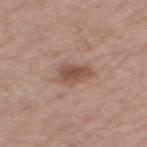notes: total-body-photography surveillance lesion; no biopsy | anatomic site: the left thigh | lighting: white-light illumination | image source: total-body-photography crop, ~15 mm field of view | patient: female, in their mid-60s | automated lesion analysis: an eccentricity of roughly 0.75 and two-axis asymmetry of about 0.25; a lesion color around L≈51 a*≈20 b*≈26 in CIELAB, a lesion–skin lightness drop of about 10, and a normalized lesion–skin contrast near 7.5; a border-irregularity rating of about 2.5/10, internal color variation of about 3.5 on a 0–10 scale, and peripheral color asymmetry of about 1 | lesion size: about 3.5 mm.